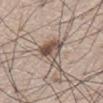| field | value |
|---|---|
| biopsy status | imaged on a skin check; not biopsied |
| patient | male, in their 80s |
| image | ~15 mm tile from a whole-body skin photo |
| anatomic site | the abdomen |